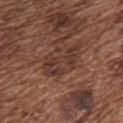Approximately 5.5 mm at its widest.
This is a white-light tile.
The lesion-visualizer software estimated a lesion area of about 10 mm² and a symmetry-axis asymmetry near 0.45. And it measured a mean CIELAB color near L≈36 a*≈20 b*≈25 and roughly 7 lightness units darker than nearby skin. The analysis additionally found border irregularity of about 7.5 on a 0–10 scale and internal color variation of about 4.5 on a 0–10 scale.
A male subject roughly 75 years of age.
Located on the chest.
This image is a 15 mm lesion crop taken from a total-body photograph.